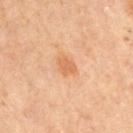Case summary:
- biopsy status · no biopsy performed (imaged during a skin exam)
- image · 15 mm crop, total-body photography
- patient · male, aged around 60
- anatomic site · the right upper arm
- illumination · cross-polarized illumination
- lesion diameter · about 2.5 mm
- TBP lesion metrics · a lesion area of about 3.5 mm² and two-axis asymmetry of about 0.35; a border-irregularity index near 3/10, internal color variation of about 1.5 on a 0–10 scale, and peripheral color asymmetry of about 0.5; a classifier nevus-likeness of about 40/100